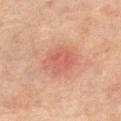Clinical impression: Recorded during total-body skin imaging; not selected for excision or biopsy. Image and clinical context: A male subject aged 73 to 77. A 15 mm close-up tile from a total-body photography series done for melanoma screening. The lesion-visualizer software estimated an average lesion color of about L≈49 a*≈24 b*≈25 (CIELAB), about 7 CIELAB-L* units darker than the surrounding skin, and a normalized lesion–skin contrast near 5.5. The software also gave a border-irregularity rating of about 2/10, internal color variation of about 3.5 on a 0–10 scale, and a peripheral color-asymmetry measure near 1. It also reported an automated nevus-likeness rating near 0 out of 100 and a lesion-detection confidence of about 100/100. On the front of the torso. Longest diameter approximately 4.5 mm. Imaged with cross-polarized lighting.